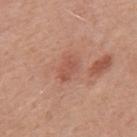{"lesion_size": {"long_diameter_mm_approx": 3.0}, "site": "mid back", "automated_metrics": {"area_mm2_approx": 4.5, "border_irregularity_0_10": 2.5, "color_variation_0_10": 2.0, "peripheral_color_asymmetry": 0.5}, "image": {"source": "total-body photography crop", "field_of_view_mm": 15}, "patient": {"sex": "male", "age_approx": 50}}